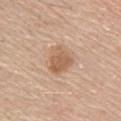The lesion was tiled from a total-body skin photograph and was not biopsied.
A male patient, aged around 70.
This image is a 15 mm lesion crop taken from a total-body photograph.
Located on the chest.
Approximately 3.5 mm at its widest.
The lesion-visualizer software estimated a lesion color around L≈59 a*≈19 b*≈33 in CIELAB, about 10 CIELAB-L* units darker than the surrounding skin, and a lesion-to-skin contrast of about 7 (normalized; higher = more distinct). It also reported a border-irregularity index near 2.5/10 and internal color variation of about 3 on a 0–10 scale. It also reported an automated nevus-likeness rating near 50 out of 100.
Captured under white-light illumination.